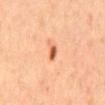This lesion was catalogued during total-body skin photography and was not selected for biopsy. A female patient roughly 65 years of age. Imaged with cross-polarized lighting. A lesion tile, about 15 mm wide, cut from a 3D total-body photograph. Located on the back.